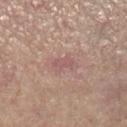The lesion was tiled from a total-body skin photograph and was not biopsied. The patient is a female roughly 65 years of age. The lesion is on the right lower leg. A 15 mm close-up tile from a total-body photography series done for melanoma screening.